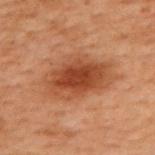A male patient aged around 70.
This image is a 15 mm lesion crop taken from a total-body photograph.
On the upper back.
The lesion-visualizer software estimated a classifier nevus-likeness of about 90/100 and a detector confidence of about 100 out of 100 that the crop contains a lesion.